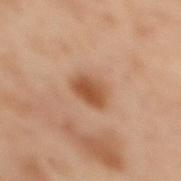<record>
  <patient>
    <sex>female</sex>
    <age_approx>55</age_approx>
  </patient>
  <automated_metrics>
    <area_mm2_approx>7.0</area_mm2_approx>
    <eccentricity>0.6</eccentricity>
    <cielab_L>43</cielab_L>
    <cielab_a>19</cielab_a>
    <cielab_b>30</cielab_b>
    <vs_skin_contrast_norm>8.5</vs_skin_contrast_norm>
    <border_irregularity_0_10>2.0</border_irregularity_0_10>
    <color_variation_0_10>3.0</color_variation_0_10>
  </automated_metrics>
  <lesion_size>
    <long_diameter_mm_approx>3.0</long_diameter_mm_approx>
  </lesion_size>
  <site>upper back</site>
  <image>
    <source>total-body photography crop</source>
    <field_of_view_mm>15</field_of_view_mm>
  </image>
  <lighting>cross-polarized</lighting>
</record>